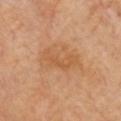The lesion was tiled from a total-body skin photograph and was not biopsied.